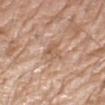This lesion was catalogued during total-body skin photography and was not selected for biopsy. The lesion is on the right upper arm. The patient is a male aged around 80. Cropped from a whole-body photographic skin survey; the tile spans about 15 mm.